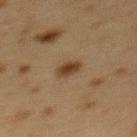<case>
  <biopsy_status>not biopsied; imaged during a skin examination</biopsy_status>
  <lighting>cross-polarized</lighting>
  <patient>
    <sex>female</sex>
    <age_approx>40</age_approx>
  </patient>
  <site>mid back</site>
  <automated_metrics>
    <cielab_L>33</cielab_L>
    <cielab_a>15</cielab_a>
    <cielab_b>27</cielab_b>
    <vs_skin_darker_L>9.0</vs_skin_darker_L>
    <vs_skin_contrast_norm>9.5</vs_skin_contrast_norm>
    <border_irregularity_0_10>2.0</border_irregularity_0_10>
    <color_variation_0_10>2.5</color_variation_0_10>
    <peripheral_color_asymmetry>1.0</peripheral_color_asymmetry>
    <nevus_likeness_0_100>100</nevus_likeness_0_100>
  </automated_metrics>
  <image>
    <source>total-body photography crop</source>
    <field_of_view_mm>15</field_of_view_mm>
  </image>
</case>